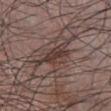– notes — imaged on a skin check; not biopsied
– image source — total-body-photography crop, ~15 mm field of view
– anatomic site — the chest
– diameter — ≈5.5 mm
– image-analysis metrics — a lesion color around L≈38 a*≈16 b*≈19 in CIELAB, roughly 9 lightness units darker than nearby skin, and a lesion-to-skin contrast of about 8 (normalized; higher = more distinct); a lesion-detection confidence of about 55/100
– subject — male, aged approximately 40